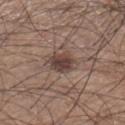workup — imaged on a skin check; not biopsied | location — the right lower leg | TBP lesion metrics — a lesion area of about 6.5 mm²; a lesion color around L≈41 a*≈16 b*≈21 in CIELAB, about 12 CIELAB-L* units darker than the surrounding skin, and a normalized lesion–skin contrast near 9.5; a border-irregularity rating of about 2.5/10 and a color-variation rating of about 3/10; a detector confidence of about 100 out of 100 that the crop contains a lesion | subject — male, aged around 35 | illumination — white-light | image — total-body-photography crop, ~15 mm field of view.